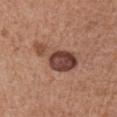workup: total-body-photography surveillance lesion; no biopsy
patient: male, about 65 years old
body site: the front of the torso
lesion diameter: about 6.5 mm
acquisition: ~15 mm tile from a whole-body skin photo